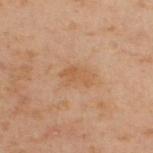{"biopsy_status": "not biopsied; imaged during a skin examination", "lighting": "cross-polarized", "image": {"source": "total-body photography crop", "field_of_view_mm": 15}, "site": "upper back", "patient": {"sex": "male", "age_approx": 50}}